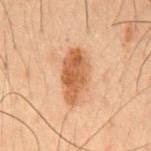This lesion was catalogued during total-body skin photography and was not selected for biopsy. A male patient roughly 50 years of age. On the chest. This image is a 15 mm lesion crop taken from a total-body photograph.